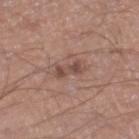Imaged during a routine full-body skin examination; the lesion was not biopsied and no histopathology is available.
Automated image analysis of the tile measured a lesion–skin lightness drop of about 9 and a lesion-to-skin contrast of about 7 (normalized; higher = more distinct). And it measured a classifier nevus-likeness of about 0/100 and a lesion-detection confidence of about 100/100.
Imaged with white-light lighting.
The lesion is located on the right lower leg.
Measured at roughly 3 mm in maximum diameter.
A male patient aged approximately 55.
A 15 mm close-up tile from a total-body photography series done for melanoma screening.